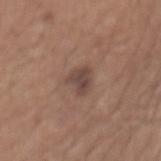Recorded during total-body skin imaging; not selected for excision or biopsy.
The lesion-visualizer software estimated a lesion area of about 5.5 mm², an outline eccentricity of about 0.65 (0 = round, 1 = elongated), and a symmetry-axis asymmetry near 0.35. The software also gave a border-irregularity rating of about 3.5/10 and a color-variation rating of about 2.5/10.
The subject is a male in their mid-60s.
Located on the mid back.
Longest diameter approximately 3 mm.
A roughly 15 mm field-of-view crop from a total-body skin photograph.
The tile uses white-light illumination.